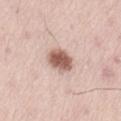Acquisition and patient details:
A male subject aged approximately 55. The lesion is located on the left thigh. A close-up tile cropped from a whole-body skin photograph, about 15 mm across. Longest diameter approximately 3 mm. This is a white-light tile.